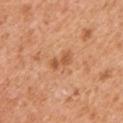* workup: total-body-photography surveillance lesion; no biopsy
* automated lesion analysis: a footprint of about 3.5 mm² and an outline eccentricity of about 0.9 (0 = round, 1 = elongated); a border-irregularity index near 4/10, a color-variation rating of about 0.5/10, and radial color variation of about 0; a nevus-likeness score of about 25/100 and lesion-presence confidence of about 100/100
* lesion size: ~2.5 mm (longest diameter)
* patient: male, aged approximately 55
* image source: ~15 mm tile from a whole-body skin photo
* site: the chest